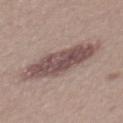| feature | finding |
|---|---|
| biopsy status | catalogued during a skin exam; not biopsied |
| automated lesion analysis | a within-lesion color-variation index near 4/10 and peripheral color asymmetry of about 1.5; an automated nevus-likeness rating near 35 out of 100 and lesion-presence confidence of about 100/100 |
| image source | 15 mm crop, total-body photography |
| illumination | white-light illumination |
| location | the mid back |
| subject | male, roughly 55 years of age |